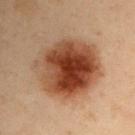Q: Is there a histopathology result?
A: catalogued during a skin exam; not biopsied
Q: Where on the body is the lesion?
A: the left upper arm
Q: What is the imaging modality?
A: ~15 mm tile from a whole-body skin photo
Q: Who is the patient?
A: male, in their mid- to late 50s